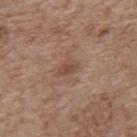Assessment: No biopsy was performed on this lesion — it was imaged during a full skin examination and was not determined to be concerning. Context: A 15 mm close-up extracted from a 3D total-body photography capture. A male subject, approximately 70 years of age. About 2.5 mm across. From the mid back. Automated image analysis of the tile measured a lesion area of about 3.5 mm² and a shape eccentricity near 0.8. Captured under white-light illumination.